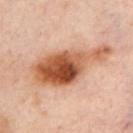Part of a total-body skin-imaging series; this lesion was reviewed on a skin check and was not flagged for biopsy. The patient is a female aged approximately 55. This image is a 15 mm lesion crop taken from a total-body photograph. The lesion's longest dimension is about 9 mm. This is a cross-polarized tile. On the chest.